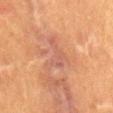| key | value |
|---|---|
| patient | male, aged approximately 50 |
| image-analysis metrics | a lesion area of about 6 mm², an outline eccentricity of about 0.8 (0 = round, 1 = elongated), and a shape-asymmetry score of about 0.7 (0 = symmetric); a mean CIELAB color near L≈47 a*≈21 b*≈25 and about 5 CIELAB-L* units darker than the surrounding skin; a border-irregularity rating of about 8.5/10, a color-variation rating of about 2/10, and radial color variation of about 0.5; an automated nevus-likeness rating near 0 out of 100 |
| location | the back |
| illumination | cross-polarized |
| diameter | ~4 mm (longest diameter) |
| image | total-body-photography crop, ~15 mm field of view |